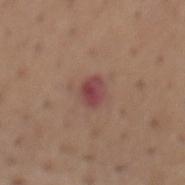The lesion was tiled from a total-body skin photograph and was not biopsied. Cropped from a whole-body photographic skin survey; the tile spans about 15 mm. The total-body-photography lesion software estimated two-axis asymmetry of about 0.15. The analysis additionally found an automated nevus-likeness rating near 0 out of 100. Imaged with white-light lighting. The lesion is located on the mid back. The recorded lesion diameter is about 3 mm. A male subject, aged around 65.